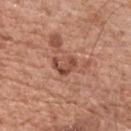The lesion was tiled from a total-body skin photograph and was not biopsied. The patient is a male about 70 years old. The lesion's longest dimension is about 3 mm. A region of skin cropped from a whole-body photographic capture, roughly 15 mm wide. The lesion is located on the chest.